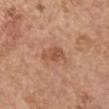Clinical impression: Recorded during total-body skin imaging; not selected for excision or biopsy. Background: This is a white-light tile. The lesion is on the left upper arm. The patient is a female aged approximately 65. A close-up tile cropped from a whole-body skin photograph, about 15 mm across. Longest diameter approximately 3 mm. An algorithmic analysis of the crop reported a footprint of about 5 mm², an outline eccentricity of about 0.8 (0 = round, 1 = elongated), and a shape-asymmetry score of about 0.3 (0 = symmetric). The analysis additionally found a lesion–skin lightness drop of about 9. The software also gave a border-irregularity index near 3/10 and a color-variation rating of about 2/10.